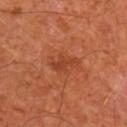Impression: The lesion was tiled from a total-body skin photograph and was not biopsied. Acquisition and patient details: The recorded lesion diameter is about 3 mm. A roughly 15 mm field-of-view crop from a total-body skin photograph. An algorithmic analysis of the crop reported a shape eccentricity near 0.85 and a symmetry-axis asymmetry near 0.4. And it measured a mean CIELAB color near L≈40 a*≈29 b*≈36, a lesion–skin lightness drop of about 7, and a lesion-to-skin contrast of about 6 (normalized; higher = more distinct). The analysis additionally found border irregularity of about 4.5 on a 0–10 scale, a color-variation rating of about 1/10, and a peripheral color-asymmetry measure near 0. The analysis additionally found an automated nevus-likeness rating near 10 out of 100 and a detector confidence of about 100 out of 100 that the crop contains a lesion. Located on the right upper arm. The subject is a male aged 63–67.